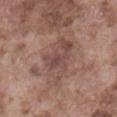Q: Was a biopsy performed?
A: imaged on a skin check; not biopsied
Q: What is the anatomic site?
A: the abdomen
Q: Who is the patient?
A: male, aged 73 to 77
Q: How was the tile lit?
A: white-light
Q: How large is the lesion?
A: ~6.5 mm (longest diameter)
Q: How was this image acquired?
A: total-body-photography crop, ~15 mm field of view